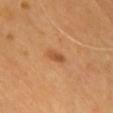Captured during whole-body skin photography for melanoma surveillance; the lesion was not biopsied.
A 15 mm close-up tile from a total-body photography series done for melanoma screening.
A male subject approximately 65 years of age.
The lesion is on the front of the torso.
The lesion-visualizer software estimated a border-irregularity rating of about 3.5/10 and peripheral color asymmetry of about 1.
Captured under cross-polarized illumination.